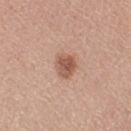notes = total-body-photography surveillance lesion; no biopsy.